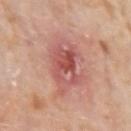Imaged during a routine full-body skin examination; the lesion was not biopsied and no histopathology is available. An algorithmic analysis of the crop reported a mean CIELAB color near L≈56 a*≈27 b*≈26 and roughly 11 lightness units darker than nearby skin. Located on the left upper arm. A male subject, roughly 75 years of age. This is a white-light tile. This image is a 15 mm lesion crop taken from a total-body photograph. The recorded lesion diameter is about 6.5 mm.